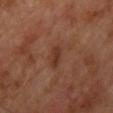Captured under cross-polarized illumination. Longest diameter approximately 2.5 mm. The lesion is located on the front of the torso. The subject is a male about 65 years old. A close-up tile cropped from a whole-body skin photograph, about 15 mm across.